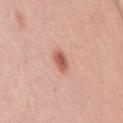Q: Was a biopsy performed?
A: no biopsy performed (imaged during a skin exam)
Q: What kind of image is this?
A: total-body-photography crop, ~15 mm field of view
Q: What is the anatomic site?
A: the right thigh
Q: How large is the lesion?
A: ~3 mm (longest diameter)
Q: What are the patient's age and sex?
A: female, approximately 20 years of age